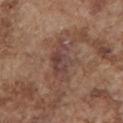Recorded during total-body skin imaging; not selected for excision or biopsy.
The tile uses white-light illumination.
A male patient in their mid- to late 70s.
An algorithmic analysis of the crop reported a mean CIELAB color near L≈42 a*≈19 b*≈22 and roughly 8 lightness units darker than nearby skin. It also reported border irregularity of about 4.5 on a 0–10 scale and a color-variation rating of about 4.5/10. It also reported an automated nevus-likeness rating near 0 out of 100 and lesion-presence confidence of about 90/100.
Located on the chest.
A roughly 15 mm field-of-view crop from a total-body skin photograph.